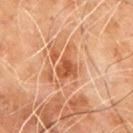The patient is a male aged 58 to 62.
The lesion is on the upper back.
Automated image analysis of the tile measured a lesion area of about 6 mm², an outline eccentricity of about 0.8 (0 = round, 1 = elongated), and two-axis asymmetry of about 0.45. It also reported internal color variation of about 3 on a 0–10 scale and peripheral color asymmetry of about 1.
Imaged with cross-polarized lighting.
This image is a 15 mm lesion crop taken from a total-body photograph.